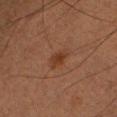No biopsy was performed on this lesion — it was imaged during a full skin examination and was not determined to be concerning. Imaged with cross-polarized lighting. On the left upper arm. Cropped from a total-body skin-imaging series; the visible field is about 15 mm. Approximately 3 mm at its widest. An algorithmic analysis of the crop reported a mean CIELAB color near L≈29 a*≈18 b*≈26, about 6 CIELAB-L* units darker than the surrounding skin, and a normalized lesion–skin contrast near 7. A male patient in their mid- to late 70s.